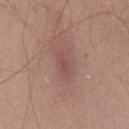Imaged during a routine full-body skin examination; the lesion was not biopsied and no histopathology is available.
Automated tile analysis of the lesion measured a lesion color around L≈50 a*≈22 b*≈21 in CIELAB. It also reported a border-irregularity index near 2/10, a within-lesion color-variation index near 2/10, and a peripheral color-asymmetry measure near 0.5.
Imaged with white-light lighting.
Located on the chest.
A male patient, aged 48–52.
The lesion's longest dimension is about 3.5 mm.
A close-up tile cropped from a whole-body skin photograph, about 15 mm across.